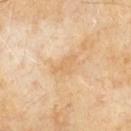No biopsy was performed on this lesion — it was imaged during a full skin examination and was not determined to be concerning.
This is a cross-polarized tile.
A male subject aged approximately 60.
Located on the mid back.
A region of skin cropped from a whole-body photographic capture, roughly 15 mm wide.